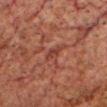TBP lesion metrics = an area of roughly 3 mm² and an eccentricity of roughly 0.85; a border-irregularity rating of about 3.5/10, a within-lesion color-variation index near 1/10, and peripheral color asymmetry of about 0.5; a classifier nevus-likeness of about 0/100 and lesion-presence confidence of about 65/100 | subject = male, roughly 60 years of age | image = 15 mm crop, total-body photography | lesion size = ~2.5 mm (longest diameter) | site = the head or neck.